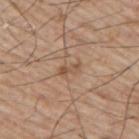* subject: male, aged around 65
* TBP lesion metrics: a nevus-likeness score of about 0/100 and a detector confidence of about 100 out of 100 that the crop contains a lesion
* anatomic site: the right upper arm
* illumination: white-light
* lesion diameter: about 2.5 mm
* image: total-body-photography crop, ~15 mm field of view